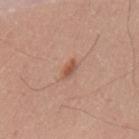biopsy_status: not biopsied; imaged during a skin examination
lighting: white-light
patient:
  sex: male
  age_approx: 60
automated_metrics:
  cielab_L: 54
  cielab_a: 22
  cielab_b: 31
  border_irregularity_0_10: 2.5
  color_variation_0_10: 1.5
  peripheral_color_asymmetry: 0.5
  nevus_likeness_0_100: 80
  lesion_detection_confidence_0_100: 100
image:
  source: total-body photography crop
  field_of_view_mm: 15
site: mid back